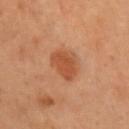Assessment:
No biopsy was performed on this lesion — it was imaged during a full skin examination and was not determined to be concerning.
Background:
This image is a 15 mm lesion crop taken from a total-body photograph. The lesion is located on the front of the torso. The lesion's longest dimension is about 4 mm. A male subject approximately 55 years of age. This is a cross-polarized tile.